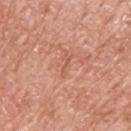Assessment: No biopsy was performed on this lesion — it was imaged during a full skin examination and was not determined to be concerning. Clinical summary: A male patient aged around 60. A lesion tile, about 15 mm wide, cut from a 3D total-body photograph. Approximately 2.5 mm at its widest. This is a white-light tile. On the upper back.